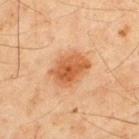biopsy status: imaged on a skin check; not biopsied
acquisition: 15 mm crop, total-body photography
subject: male, about 45 years old
body site: the upper back